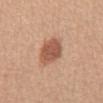Findings:
- workup — catalogued during a skin exam; not biopsied
- lesion size — about 4 mm
- site — the front of the torso
- patient — female, roughly 55 years of age
- TBP lesion metrics — a lesion area of about 10 mm² and a shape eccentricity near 0.6; a border-irregularity index near 1.5/10 and peripheral color asymmetry of about 1; an automated nevus-likeness rating near 100 out of 100 and a detector confidence of about 100 out of 100 that the crop contains a lesion
- image — ~15 mm tile from a whole-body skin photo
- tile lighting — white-light illumination